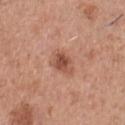{
  "biopsy_status": "not biopsied; imaged during a skin examination",
  "site": "chest",
  "lighting": "white-light",
  "patient": {
    "sex": "male",
    "age_approx": 45
  },
  "image": {
    "source": "total-body photography crop",
    "field_of_view_mm": 15
  },
  "lesion_size": {
    "long_diameter_mm_approx": 3.5
  }
}